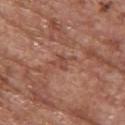Assessment: Recorded during total-body skin imaging; not selected for excision or biopsy. Context: On the upper back. Cropped from a whole-body photographic skin survey; the tile spans about 15 mm. Automated image analysis of the tile measured an area of roughly 3.5 mm², an outline eccentricity of about 0.8 (0 = round, 1 = elongated), and two-axis asymmetry of about 0.4. The analysis additionally found a lesion color around L≈47 a*≈24 b*≈28 in CIELAB, roughly 7 lightness units darker than nearby skin, and a normalized lesion–skin contrast near 5.5. It also reported an automated nevus-likeness rating near 0 out of 100 and a detector confidence of about 95 out of 100 that the crop contains a lesion. A female patient aged approximately 65.